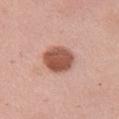Q: Was this lesion biopsied?
A: imaged on a skin check; not biopsied
Q: How was the tile lit?
A: white-light illumination
Q: What is the imaging modality?
A: ~15 mm tile from a whole-body skin photo
Q: Who is the patient?
A: female, aged around 50
Q: Automated lesion metrics?
A: an average lesion color of about L≈54 a*≈24 b*≈29 (CIELAB), a lesion–skin lightness drop of about 16, and a lesion-to-skin contrast of about 10.5 (normalized; higher = more distinct); a border-irregularity index near 1/10 and internal color variation of about 3.5 on a 0–10 scale
Q: What is the lesion's diameter?
A: ~4.5 mm (longest diameter)
Q: Lesion location?
A: the right upper arm